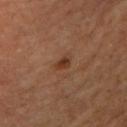  biopsy_status: not biopsied; imaged during a skin examination
  lighting: cross-polarized
  patient:
    sex: female
    age_approx: 70
  image:
    source: total-body photography crop
    field_of_view_mm: 15
  lesion_size:
    long_diameter_mm_approx: 2.0
  site: right upper arm